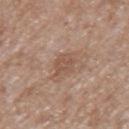This is a white-light tile.
A male subject, aged 63–67.
A 15 mm close-up tile from a total-body photography series done for melanoma screening.
Automated image analysis of the tile measured an eccentricity of roughly 0.55 and two-axis asymmetry of about 0.25. The analysis additionally found a lesion color around L≈52 a*≈19 b*≈28 in CIELAB, about 7 CIELAB-L* units darker than the surrounding skin, and a lesion-to-skin contrast of about 5.5 (normalized; higher = more distinct). The software also gave a classifier nevus-likeness of about 0/100 and a lesion-detection confidence of about 100/100.
Located on the mid back.
Approximately 2.5 mm at its widest.